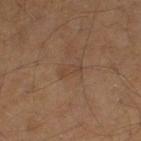Background: The subject is a male approximately 60 years of age. The lesion is located on the left thigh. A 15 mm close-up extracted from a 3D total-body photography capture.